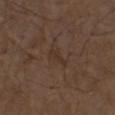* workup · catalogued during a skin exam; not biopsied
* patient · male, aged approximately 75
* anatomic site · the left thigh
* imaging modality · ~15 mm tile from a whole-body skin photo